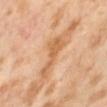Clinical impression: Part of a total-body skin-imaging series; this lesion was reviewed on a skin check and was not flagged for biopsy. Image and clinical context: On the abdomen. A 15 mm close-up extracted from a 3D total-body photography capture. A female subject, aged 53–57.